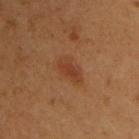Recorded during total-body skin imaging; not selected for excision or biopsy. On the chest. A male patient approximately 50 years of age. A 15 mm crop from a total-body photograph taken for skin-cancer surveillance. About 3.5 mm across.